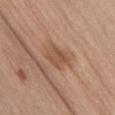workup = no biopsy performed (imaged during a skin exam) | image = ~15 mm tile from a whole-body skin photo | automated lesion analysis = a mean CIELAB color near L≈52 a*≈20 b*≈30 and about 8 CIELAB-L* units darker than the surrounding skin; internal color variation of about 3.5 on a 0–10 scale and radial color variation of about 1.5 | patient = male, aged approximately 65 | tile lighting = white-light illumination | diameter = ≈3 mm | anatomic site = the abdomen.